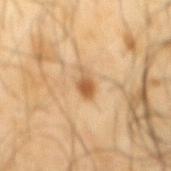This lesion was catalogued during total-body skin photography and was not selected for biopsy.
Captured under cross-polarized illumination.
A male subject, aged 63 to 67.
The total-body-photography lesion software estimated a shape eccentricity near 0.8 and two-axis asymmetry of about 0.4. And it measured border irregularity of about 4 on a 0–10 scale, a within-lesion color-variation index near 3/10, and peripheral color asymmetry of about 1. And it measured a classifier nevus-likeness of about 95/100 and lesion-presence confidence of about 100/100.
Cropped from a whole-body photographic skin survey; the tile spans about 15 mm.
The lesion's longest dimension is about 3 mm.
Located on the mid back.